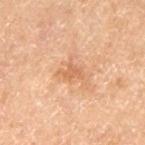notes: catalogued during a skin exam; not biopsied
tile lighting: cross-polarized illumination
body site: the left thigh
imaging modality: ~15 mm tile from a whole-body skin photo
diameter: ~3 mm (longest diameter)
subject: male, aged 68 to 72